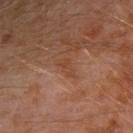Captured during whole-body skin photography for melanoma surveillance; the lesion was not biopsied. Located on the left arm. A region of skin cropped from a whole-body photographic capture, roughly 15 mm wide. The patient is a male aged around 30.